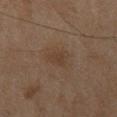| key | value |
|---|---|
| notes | total-body-photography surveillance lesion; no biopsy |
| patient | female, roughly 60 years of age |
| tile lighting | cross-polarized illumination |
| body site | the leg |
| image | ~15 mm tile from a whole-body skin photo |
| diameter | about 3 mm |
| image-analysis metrics | an area of roughly 5.5 mm², an eccentricity of roughly 0.35, and a symmetry-axis asymmetry near 0.3; a border-irregularity index near 2.5/10, a color-variation rating of about 1.5/10, and a peripheral color-asymmetry measure near 0.5; a nevus-likeness score of about 15/100 |